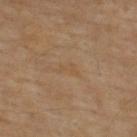| field | value |
|---|---|
| workup | no biopsy performed (imaged during a skin exam) |
| image | 15 mm crop, total-body photography |
| subject | male, aged approximately 65 |
| illumination | cross-polarized |
| size | about 2.5 mm |
| body site | the upper back |